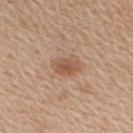Clinical impression:
This lesion was catalogued during total-body skin photography and was not selected for biopsy.
Clinical summary:
A male subject, approximately 60 years of age. Measured at roughly 3.5 mm in maximum diameter. Cropped from a total-body skin-imaging series; the visible field is about 15 mm. Automated tile analysis of the lesion measured a footprint of about 5.5 mm², an outline eccentricity of about 0.8 (0 = round, 1 = elongated), and a symmetry-axis asymmetry near 0.2. The software also gave a lesion color around L≈54 a*≈20 b*≈32 in CIELAB, roughly 9 lightness units darker than nearby skin, and a normalized lesion–skin contrast near 7. The analysis additionally found border irregularity of about 2 on a 0–10 scale, a within-lesion color-variation index near 3.5/10, and a peripheral color-asymmetry measure near 1. The lesion is on the right upper arm. The tile uses white-light illumination.